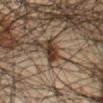Automated image analysis of the tile measured a shape-asymmetry score of about 0.3 (0 = symmetric). And it measured a mean CIELAB color near L≈33 a*≈16 b*≈26, about 14 CIELAB-L* units darker than the surrounding skin, and a normalized lesion–skin contrast near 12.5. And it measured border irregularity of about 3.5 on a 0–10 scale, internal color variation of about 3 on a 0–10 scale, and a peripheral color-asymmetry measure near 1. This is a cross-polarized tile. The lesion is located on the front of the torso. The lesion's longest dimension is about 3.5 mm. A 15 mm close-up tile from a total-body photography series done for melanoma screening. The subject is a male aged around 45.